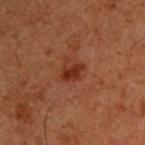Clinical impression: This lesion was catalogued during total-body skin photography and was not selected for biopsy. Background: A roughly 15 mm field-of-view crop from a total-body skin photograph. Imaged with cross-polarized lighting. A male patient, aged around 60. Longest diameter approximately 3 mm. From the upper back.